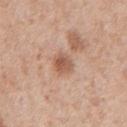Q: Was a biopsy performed?
A: imaged on a skin check; not biopsied
Q: What is the anatomic site?
A: the mid back
Q: What lighting was used for the tile?
A: white-light
Q: Lesion size?
A: about 3 mm
Q: How was this image acquired?
A: ~15 mm tile from a whole-body skin photo
Q: Patient demographics?
A: male, in their mid-60s